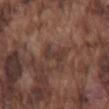Q: Was a biopsy performed?
A: catalogued during a skin exam; not biopsied
Q: Who is the patient?
A: male, aged 73 to 77
Q: How large is the lesion?
A: ≈3 mm
Q: Illumination type?
A: white-light illumination
Q: What is the anatomic site?
A: the mid back
Q: What is the imaging modality?
A: total-body-photography crop, ~15 mm field of view